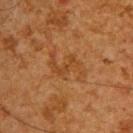Case summary:
– workup · catalogued during a skin exam; not biopsied
– site · the upper back
– patient · male, aged approximately 65
– diameter · about 4.5 mm
– TBP lesion metrics · an average lesion color of about L≈36 a*≈20 b*≈33 (CIELAB) and a normalized lesion–skin contrast near 5; border irregularity of about 8.5 on a 0–10 scale, a within-lesion color-variation index near 2/10, and peripheral color asymmetry of about 0.5; an automated nevus-likeness rating near 0 out of 100 and a lesion-detection confidence of about 100/100
– tile lighting · cross-polarized
– imaging modality · ~15 mm crop, total-body skin-cancer survey